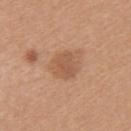• anatomic site: the left upper arm
• lesion diameter: ≈3 mm
• acquisition: ~15 mm tile from a whole-body skin photo
• subject: female, aged 18–22
• lighting: white-light illumination